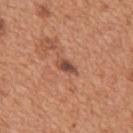biopsy status: total-body-photography surveillance lesion; no biopsy
image: ~15 mm crop, total-body skin-cancer survey
location: the mid back
lesion diameter: about 2.5 mm
automated lesion analysis: a footprint of about 3 mm²; a lesion color around L≈47 a*≈23 b*≈28 in CIELAB and a normalized lesion–skin contrast near 9.5; a border-irregularity index near 2.5/10 and radial color variation of about 1
patient: male, aged approximately 65